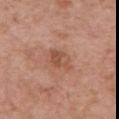Q: Automated lesion metrics?
A: a footprint of about 6.5 mm², a shape eccentricity near 0.65, and two-axis asymmetry of about 0.2
Q: Who is the patient?
A: male, aged approximately 80
Q: Lesion location?
A: the chest
Q: How was the tile lit?
A: white-light illumination
Q: What is the lesion's diameter?
A: ≈3 mm
Q: What is the imaging modality?
A: total-body-photography crop, ~15 mm field of view